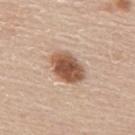Clinical impression:
Imaged during a routine full-body skin examination; the lesion was not biopsied and no histopathology is available.
Background:
On the upper back. A male subject, about 60 years old. Approximately 4.5 mm at its widest. Cropped from a whole-body photographic skin survey; the tile spans about 15 mm.